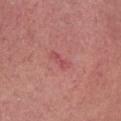– notes — no biopsy performed (imaged during a skin exam)
– subject — female, aged approximately 65
– image — total-body-photography crop, ~15 mm field of view
– body site — the chest
– image-analysis metrics — border irregularity of about 3.5 on a 0–10 scale, a color-variation rating of about 0/10, and peripheral color asymmetry of about 0
– diameter — ~3 mm (longest diameter)